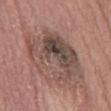Impression:
No biopsy was performed on this lesion — it was imaged during a full skin examination and was not determined to be concerning.
Clinical summary:
A lesion tile, about 15 mm wide, cut from a 3D total-body photograph. On the abdomen. A male patient, approximately 75 years of age.